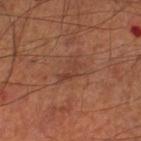| key | value |
|---|---|
| biopsy status | imaged on a skin check; not biopsied |
| tile lighting | cross-polarized |
| location | the left lower leg |
| lesion diameter | ~3.5 mm (longest diameter) |
| image source | ~15 mm tile from a whole-body skin photo |
| subject | male, aged approximately 70 |
| automated lesion analysis | a footprint of about 4.5 mm², an eccentricity of roughly 0.85, and two-axis asymmetry of about 0.5 |